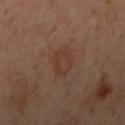| feature | finding |
|---|---|
| follow-up | catalogued during a skin exam; not biopsied |
| image | ~15 mm tile from a whole-body skin photo |
| subject | female, in their 40s |
| site | the left arm |